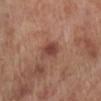Assessment:
The lesion was tiled from a total-body skin photograph and was not biopsied.
Clinical summary:
Captured under white-light illumination. Located on the left lower leg. A male subject aged around 70. Cropped from a total-body skin-imaging series; the visible field is about 15 mm. The recorded lesion diameter is about 2.5 mm.